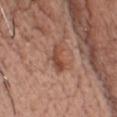Notes:
- workup — imaged on a skin check; not biopsied
- TBP lesion metrics — a lesion area of about 5 mm², an eccentricity of roughly 0.85, and two-axis asymmetry of about 0.35; a lesion–skin lightness drop of about 10 and a normalized border contrast of about 7.5; internal color variation of about 3.5 on a 0–10 scale; a nevus-likeness score of about 15/100 and a detector confidence of about 100 out of 100 that the crop contains a lesion
- image — 15 mm crop, total-body photography
- anatomic site — the chest
- diameter — ≈3.5 mm
- lighting — white-light illumination
- subject — male, aged around 60